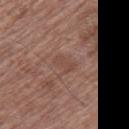Imaged during a routine full-body skin examination; the lesion was not biopsied and no histopathology is available.
This is a white-light tile.
The patient is a male aged approximately 75.
A lesion tile, about 15 mm wide, cut from a 3D total-body photograph.
The lesion is located on the right thigh.
The lesion-visualizer software estimated a footprint of about 3.5 mm² and an eccentricity of roughly 0.85. It also reported a lesion color around L≈46 a*≈20 b*≈25 in CIELAB, a lesion–skin lightness drop of about 6, and a normalized lesion–skin contrast near 5.